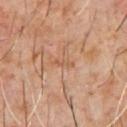Q: Is there a histopathology result?
A: catalogued during a skin exam; not biopsied
Q: What is the anatomic site?
A: the chest
Q: What kind of image is this?
A: total-body-photography crop, ~15 mm field of view
Q: What are the patient's age and sex?
A: male, aged 58–62
Q: Lesion size?
A: ~2.5 mm (longest diameter)